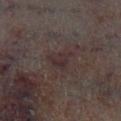A male subject approximately 60 years of age.
Captured under cross-polarized illumination.
Longest diameter approximately 3.5 mm.
The lesion is on the left lower leg.
A 15 mm close-up tile from a total-body photography series done for melanoma screening.